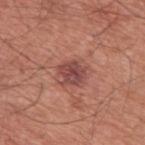– notes: total-body-photography surveillance lesion; no biopsy
– site: the upper back
– lighting: white-light illumination
– diameter: ~3 mm (longest diameter)
– patient: male, aged around 60
– automated lesion analysis: a footprint of about 5.5 mm² and a shape eccentricity near 0.45; a classifier nevus-likeness of about 10/100 and a lesion-detection confidence of about 100/100
– imaging modality: 15 mm crop, total-body photography